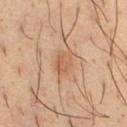Clinical impression: Recorded during total-body skin imaging; not selected for excision or biopsy. Clinical summary: A male subject aged 33–37. This is a cross-polarized tile. Cropped from a total-body skin-imaging series; the visible field is about 15 mm. Measured at roughly 3 mm in maximum diameter. Located on the chest.